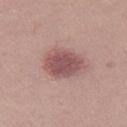Notes:
* biopsy status: catalogued during a skin exam; not biopsied
* anatomic site: the right thigh
* automated lesion analysis: a footprint of about 13 mm² and a shape eccentricity near 0.6; a border-irregularity rating of about 1.5/10, internal color variation of about 3 on a 0–10 scale, and a peripheral color-asymmetry measure near 1; a nevus-likeness score of about 85/100 and a detector confidence of about 100 out of 100 that the crop contains a lesion
* lighting: white-light
* patient: female, aged 18 to 22
* imaging modality: total-body-photography crop, ~15 mm field of view